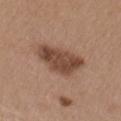biopsy_status: not biopsied; imaged during a skin examination
lighting: white-light
image:
  source: total-body photography crop
  field_of_view_mm: 15
lesion_size:
  long_diameter_mm_approx: 5.5
site: right upper arm
automated_metrics:
  area_mm2_approx: 15.0
  eccentricity: 0.75
  shape_asymmetry: 0.3
  border_irregularity_0_10: 3.5
  color_variation_0_10: 3.5
patient:
  sex: female
  age_approx: 40